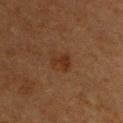<case>
  <biopsy_status>not biopsied; imaged during a skin examination</biopsy_status>
</case>